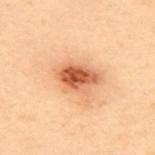Q: Is there a histopathology result?
A: catalogued during a skin exam; not biopsied
Q: What is the lesion's diameter?
A: ≈5 mm
Q: Patient demographics?
A: male, in their mid- to late 50s
Q: Automated lesion metrics?
A: a footprint of about 11 mm²; an average lesion color of about L≈50 a*≈23 b*≈33 (CIELAB), about 13 CIELAB-L* units darker than the surrounding skin, and a lesion-to-skin contrast of about 9.5 (normalized; higher = more distinct)
Q: What lighting was used for the tile?
A: cross-polarized illumination
Q: What is the anatomic site?
A: the back
Q: What kind of image is this?
A: ~15 mm crop, total-body skin-cancer survey